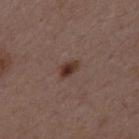Assessment: The lesion was photographed on a routine skin check and not biopsied; there is no pathology result. Clinical summary: The tile uses white-light illumination. A male subject aged 48–52. About 2.5 mm across. The lesion is located on the mid back. A 15 mm crop from a total-body photograph taken for skin-cancer surveillance.